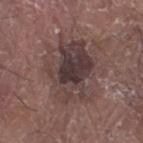Q: Is there a histopathology result?
A: catalogued during a skin exam; not biopsied
Q: Automated lesion metrics?
A: a lesion area of about 23 mm², an outline eccentricity of about 0.8 (0 = round, 1 = elongated), and a symmetry-axis asymmetry near 0.3; an automated nevus-likeness rating near 60 out of 100 and a detector confidence of about 100 out of 100 that the crop contains a lesion
Q: What kind of image is this?
A: ~15 mm crop, total-body skin-cancer survey
Q: Where on the body is the lesion?
A: the arm
Q: What are the patient's age and sex?
A: male, in their 80s